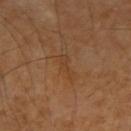{
  "lighting": "cross-polarized",
  "image": {
    "source": "total-body photography crop",
    "field_of_view_mm": 15
  },
  "lesion_size": {
    "long_diameter_mm_approx": 3.0
  },
  "automated_metrics": {
    "area_mm2_approx": 3.5,
    "eccentricity": 0.9,
    "shape_asymmetry": 0.35,
    "cielab_L": 41,
    "cielab_a": 20,
    "cielab_b": 34,
    "vs_skin_contrast_norm": 4.5
  },
  "site": "arm"
}